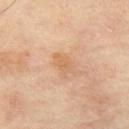{
  "biopsy_status": "not biopsied; imaged during a skin examination",
  "site": "left thigh",
  "lesion_size": {
    "long_diameter_mm_approx": 3.5
  },
  "lighting": "cross-polarized",
  "automated_metrics": {
    "area_mm2_approx": 5.5,
    "eccentricity": 0.75,
    "shape_asymmetry": 0.4,
    "border_irregularity_0_10": 4.5
  },
  "image": {
    "source": "total-body photography crop",
    "field_of_view_mm": 15
  },
  "patient": {
    "sex": "male",
    "age_approx": 65
  }
}